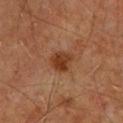Part of a total-body skin-imaging series; this lesion was reviewed on a skin check and was not flagged for biopsy.
This image is a 15 mm lesion crop taken from a total-body photograph.
A male subject roughly 60 years of age.
Captured under cross-polarized illumination.
An algorithmic analysis of the crop reported a border-irregularity index near 2/10 and a peripheral color-asymmetry measure near 1. The analysis additionally found a detector confidence of about 100 out of 100 that the crop contains a lesion.
Located on the mid back.